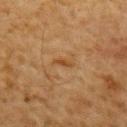tile lighting — cross-polarized; anatomic site — the mid back; image source — ~15 mm tile from a whole-body skin photo; subject — male, about 60 years old; lesion diameter — about 2.5 mm.